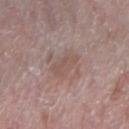| key | value |
|---|---|
| anatomic site | the right forearm |
| patient | female, aged approximately 65 |
| lesion size | ~3.5 mm (longest diameter) |
| illumination | white-light |
| acquisition | ~15 mm crop, total-body skin-cancer survey |